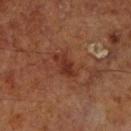Clinical impression: Captured during whole-body skin photography for melanoma surveillance; the lesion was not biopsied.